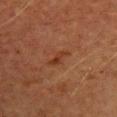<record>
  <biopsy_status>not biopsied; imaged during a skin examination</biopsy_status>
  <image>
    <source>total-body photography crop</source>
    <field_of_view_mm>15</field_of_view_mm>
  </image>
  <patient>
    <sex>female</sex>
    <age_approx>50</age_approx>
  </patient>
  <lesion_size>
    <long_diameter_mm_approx>3.0</long_diameter_mm_approx>
  </lesion_size>
  <automated_metrics>
    <eccentricity>0.9</eccentricity>
    <shape_asymmetry>0.45</shape_asymmetry>
    <vs_skin_darker_L>6.0</vs_skin_darker_L>
    <vs_skin_contrast_norm>6.5</vs_skin_contrast_norm>
    <color_variation_0_10>0.0</color_variation_0_10>
    <peripheral_color_asymmetry>0.0</peripheral_color_asymmetry>
  </automated_metrics>
  <site>chest</site>
</record>